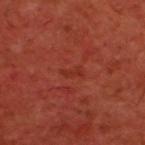Case summary:
– notes — no biopsy performed (imaged during a skin exam)
– size — ≈2.5 mm
– automated lesion analysis — a lesion color around L≈31 a*≈31 b*≈31 in CIELAB, roughly 4 lightness units darker than nearby skin, and a normalized lesion–skin contrast near 4.5; a within-lesion color-variation index near 0/10 and radial color variation of about 0; a classifier nevus-likeness of about 0/100 and a lesion-detection confidence of about 90/100
– illumination — cross-polarized illumination
– patient — male, aged 58–62
– body site — the upper back
– image source — ~15 mm crop, total-body skin-cancer survey